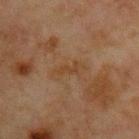This lesion was catalogued during total-body skin photography and was not selected for biopsy. This is a cross-polarized tile. A lesion tile, about 15 mm wide, cut from a 3D total-body photograph. On the chest. A male patient, aged 63–67. Approximately 3 mm at its widest.